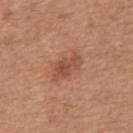Notes:
- notes — no biopsy performed (imaged during a skin exam)
- diameter — ≈4 mm
- automated lesion analysis — a lesion color around L≈50 a*≈25 b*≈31 in CIELAB and a lesion-to-skin contrast of about 6.5 (normalized; higher = more distinct); border irregularity of about 2.5 on a 0–10 scale, a within-lesion color-variation index near 3.5/10, and radial color variation of about 1
- patient — male, approximately 65 years of age
- tile lighting — white-light illumination
- location — the abdomen
- image — 15 mm crop, total-body photography